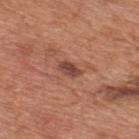biopsy status: imaged on a skin check; not biopsied | imaging modality: ~15 mm crop, total-body skin-cancer survey | subject: male, about 65 years old | tile lighting: white-light | lesion size: about 3 mm | image-analysis metrics: an average lesion color of about L≈45 a*≈23 b*≈27 (CIELAB), a lesion–skin lightness drop of about 11, and a normalized lesion–skin contrast near 9 | location: the upper back.